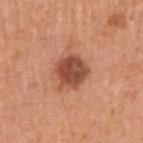Q: Was this lesion biopsied?
A: no biopsy performed (imaged during a skin exam)
Q: What is the anatomic site?
A: the right upper arm
Q: How was this image acquired?
A: ~15 mm crop, total-body skin-cancer survey
Q: Who is the patient?
A: male, aged approximately 55